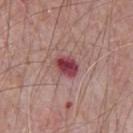The lesion was tiled from a total-body skin photograph and was not biopsied.
A male subject aged 63–67.
The tile uses white-light illumination.
On the chest.
Cropped from a total-body skin-imaging series; the visible field is about 15 mm.
An algorithmic analysis of the crop reported a shape eccentricity near 0.65 and a symmetry-axis asymmetry near 0.15. It also reported a border-irregularity rating of about 1/10, a color-variation rating of about 6/10, and peripheral color asymmetry of about 2.
Longest diameter approximately 3 mm.